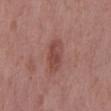Q: Is there a histopathology result?
A: no biopsy performed (imaged during a skin exam)
Q: Where on the body is the lesion?
A: the mid back
Q: What are the patient's age and sex?
A: male, aged 58 to 62
Q: Lesion size?
A: about 4 mm
Q: What is the imaging modality?
A: ~15 mm crop, total-body skin-cancer survey